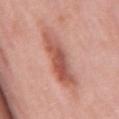A male patient in their mid- to late 50s.
Cropped from a total-body skin-imaging series; the visible field is about 15 mm.
The lesion is on the mid back.